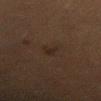<tbp_lesion>
<biopsy_status>not biopsied; imaged during a skin examination</biopsy_status>
<lesion_size>
  <long_diameter_mm_approx>3.0</long_diameter_mm_approx>
</lesion_size>
<patient>
  <sex>female</sex>
  <age_approx>50</age_approx>
</patient>
<image>
  <source>total-body photography crop</source>
  <field_of_view_mm>15</field_of_view_mm>
</image>
<site>front of the torso</site>
</tbp_lesion>